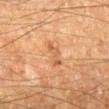<record>
<biopsy_status>not biopsied; imaged during a skin examination</biopsy_status>
<lesion_size>
  <long_diameter_mm_approx>3.0</long_diameter_mm_approx>
</lesion_size>
<patient>
  <sex>male</sex>
  <age_approx>65</age_approx>
</patient>
<site>right lower leg</site>
<image>
  <source>total-body photography crop</source>
  <field_of_view_mm>15</field_of_view_mm>
</image>
</record>